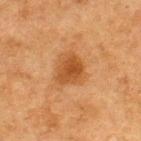| field | value |
|---|---|
| notes | catalogued during a skin exam; not biopsied |
| acquisition | ~15 mm tile from a whole-body skin photo |
| lesion diameter | about 3.5 mm |
| location | the back |
| automated lesion analysis | a color-variation rating of about 2.5/10 and peripheral color asymmetry of about 1; lesion-presence confidence of about 100/100 |
| tile lighting | cross-polarized |
| patient | male, aged 73 to 77 |